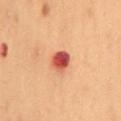Q: Was this lesion biopsied?
A: imaged on a skin check; not biopsied
Q: Automated lesion metrics?
A: an average lesion color of about L≈42 a*≈32 b*≈28 (CIELAB), a lesion–skin lightness drop of about 17, and a normalized border contrast of about 12.5; a color-variation rating of about 4.5/10 and radial color variation of about 1.5
Q: What is the imaging modality?
A: total-body-photography crop, ~15 mm field of view
Q: What are the patient's age and sex?
A: male, roughly 55 years of age
Q: Lesion location?
A: the back
Q: How large is the lesion?
A: ~2.5 mm (longest diameter)
Q: How was the tile lit?
A: cross-polarized